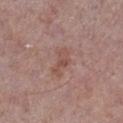Located on the right lower leg. A male patient, about 70 years old. A roughly 15 mm field-of-view crop from a total-body skin photograph.